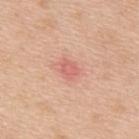Image and clinical context: Imaged with white-light lighting. On the upper back. A 15 mm close-up extracted from a 3D total-body photography capture. The recorded lesion diameter is about 2.5 mm. The subject is a male about 50 years old.